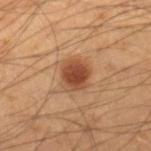No biopsy was performed on this lesion — it was imaged during a full skin examination and was not determined to be concerning. This is a cross-polarized tile. A 15 mm close-up tile from a total-body photography series done for melanoma screening. A male patient aged around 40. The lesion is located on the right lower leg. Longest diameter approximately 3.5 mm.